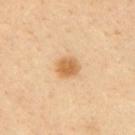Impression: No biopsy was performed on this lesion — it was imaged during a full skin examination and was not determined to be concerning. Clinical summary: A male patient in their 40s. A close-up tile cropped from a whole-body skin photograph, about 15 mm across. This is a cross-polarized tile. The lesion is on the back. Measured at roughly 2.5 mm in maximum diameter.